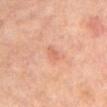Captured during whole-body skin photography for melanoma surveillance; the lesion was not biopsied. On the mid back. Measured at roughly 2.5 mm in maximum diameter. The total-body-photography lesion software estimated an eccentricity of roughly 0.9 and two-axis asymmetry of about 0.35. It also reported a border-irregularity rating of about 3.5/10, internal color variation of about 0 on a 0–10 scale, and peripheral color asymmetry of about 0. It also reported a classifier nevus-likeness of about 0/100 and a lesion-detection confidence of about 100/100. A male subject, about 65 years old. Imaged with cross-polarized lighting. A 15 mm crop from a total-body photograph taken for skin-cancer surveillance.